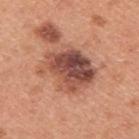– biopsy status: no biopsy performed (imaged during a skin exam)
– patient: male, roughly 45 years of age
– location: the upper back
– size: ≈6 mm
– image-analysis metrics: a lesion area of about 22 mm², a shape eccentricity near 0.65, and a symmetry-axis asymmetry near 0.3; an automated nevus-likeness rating near 15 out of 100 and a lesion-detection confidence of about 100/100
– acquisition: ~15 mm tile from a whole-body skin photo
– tile lighting: white-light